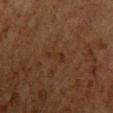| field | value |
|---|---|
| notes | catalogued during a skin exam; not biopsied |
| lesion size | ~3 mm (longest diameter) |
| site | the chest |
| illumination | cross-polarized illumination |
| subject | male, aged 63–67 |
| imaging modality | ~15 mm tile from a whole-body skin photo |